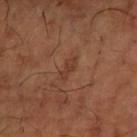Assessment:
This lesion was catalogued during total-body skin photography and was not selected for biopsy.
Background:
The subject is a male about 65 years old. The lesion-visualizer software estimated border irregularity of about 3.5 on a 0–10 scale, a within-lesion color-variation index near 1/10, and a peripheral color-asymmetry measure near 0. Measured at roughly 3 mm in maximum diameter. Imaged with cross-polarized lighting. A 15 mm close-up tile from a total-body photography series done for melanoma screening. The lesion is located on the left forearm.